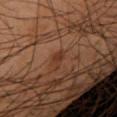Imaged during a routine full-body skin examination; the lesion was not biopsied and no histopathology is available. A roughly 15 mm field-of-view crop from a total-body skin photograph. The patient is a male roughly 55 years of age. Automated tile analysis of the lesion measured an average lesion color of about L≈28 a*≈18 b*≈25 (CIELAB), a lesion–skin lightness drop of about 6, and a normalized border contrast of about 6.5. And it measured a border-irregularity rating of about 2.5/10. The analysis additionally found a detector confidence of about 100 out of 100 that the crop contains a lesion. Imaged with cross-polarized lighting. The lesion is located on the right forearm.